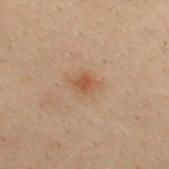  biopsy_status: not biopsied; imaged during a skin examination
  lesion_size:
    long_diameter_mm_approx: 3.0
  site: upper back
  image:
    source: total-body photography crop
    field_of_view_mm: 15
  patient:
    sex: male
    age_approx: 30